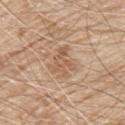Assessment:
No biopsy was performed on this lesion — it was imaged during a full skin examination and was not determined to be concerning.
Context:
Captured under white-light illumination. A male patient aged around 80. From the arm. A 15 mm close-up extracted from a 3D total-body photography capture. The recorded lesion diameter is about 3.5 mm.